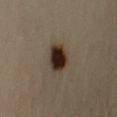Acquisition and patient details:
The lesion is located on the arm. About 3.5 mm across. The patient is a male roughly 40 years of age. This image is a 15 mm lesion crop taken from a total-body photograph. Captured under cross-polarized illumination.